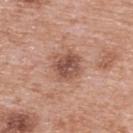| field | value |
|---|---|
| workup | imaged on a skin check; not biopsied |
| patient | female, about 60 years old |
| automated lesion analysis | a footprint of about 9.5 mm² and a symmetry-axis asymmetry near 0.2; a lesion color around L≈52 a*≈22 b*≈27 in CIELAB; a border-irregularity rating of about 2.5/10, a within-lesion color-variation index near 5/10, and peripheral color asymmetry of about 1.5; an automated nevus-likeness rating near 50 out of 100 and lesion-presence confidence of about 100/100 |
| body site | the upper back |
| diameter | ≈3.5 mm |
| image | ~15 mm tile from a whole-body skin photo |